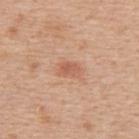Part of a total-body skin-imaging series; this lesion was reviewed on a skin check and was not flagged for biopsy. Measured at roughly 2.5 mm in maximum diameter. The patient is a female aged 48–52. A 15 mm close-up extracted from a 3D total-body photography capture. The lesion is on the upper back. An algorithmic analysis of the crop reported a border-irregularity index near 2/10, a within-lesion color-variation index near 3.5/10, and radial color variation of about 1.5. It also reported a detector confidence of about 100 out of 100 that the crop contains a lesion. Imaged with white-light lighting.